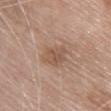biopsy_status: not biopsied; imaged during a skin examination
patient:
  sex: female
  age_approx: 75
lighting: white-light
site: chest
automated_metrics:
  cielab_L: 53
  cielab_a: 18
  cielab_b: 29
  vs_skin_darker_L: 9.0
  vs_skin_contrast_norm: 6.5
  color_variation_0_10: 3.0
  peripheral_color_asymmetry: 1.0
  nevus_likeness_0_100: 25
  lesion_detection_confidence_0_100: 100
lesion_size:
  long_diameter_mm_approx: 3.5
image:
  source: total-body photography crop
  field_of_view_mm: 15